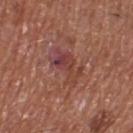Case summary:
- notes · imaged on a skin check; not biopsied
- image source · 15 mm crop, total-body photography
- tile lighting · white-light
- anatomic site · the right thigh
- patient · male, aged 73–77
- size · ≈5 mm